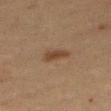Clinical summary: From the right thigh. Cropped from a total-body skin-imaging series; the visible field is about 15 mm. The patient is a female in their 40s. The total-body-photography lesion software estimated a footprint of about 4.5 mm², an outline eccentricity of about 0.9 (0 = round, 1 = elongated), and a symmetry-axis asymmetry near 0.3. The analysis additionally found an average lesion color of about L≈37 a*≈16 b*≈28 (CIELAB) and a lesion-to-skin contrast of about 8 (normalized; higher = more distinct). It also reported a border-irregularity index near 3/10, a within-lesion color-variation index near 1.5/10, and peripheral color asymmetry of about 0.5. This is a cross-polarized tile.